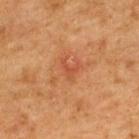Imaged during a routine full-body skin examination; the lesion was not biopsied and no histopathology is available. Approximately 2.5 mm at its widest. Cropped from a total-body skin-imaging series; the visible field is about 15 mm. The patient is a male in their mid- to late 60s. From the upper back.